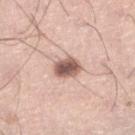follow-up: catalogued during a skin exam; not biopsied
body site: the right lower leg
lesion diameter: about 3.5 mm
tile lighting: white-light
TBP lesion metrics: a mean CIELAB color near L≈57 a*≈20 b*≈24 and a lesion-to-skin contrast of about 11 (normalized; higher = more distinct)
image: ~15 mm tile from a whole-body skin photo
patient: male, in their mid- to late 30s